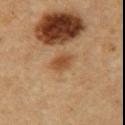No biopsy was performed on this lesion — it was imaged during a full skin examination and was not determined to be concerning.
Captured under cross-polarized illumination.
On the right upper arm.
This image is a 15 mm lesion crop taken from a total-body photograph.
The subject is a male aged 53–57.